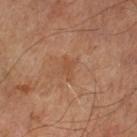Notes:
- workup: imaged on a skin check; not biopsied
- size: about 2.5 mm
- image: ~15 mm crop, total-body skin-cancer survey
- subject: male, aged around 65